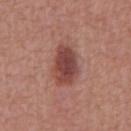Clinical impression:
Captured during whole-body skin photography for melanoma surveillance; the lesion was not biopsied.
Image and clinical context:
A 15 mm close-up tile from a total-body photography series done for melanoma screening. The recorded lesion diameter is about 5 mm. On the abdomen. Imaged with white-light lighting. A male patient, about 65 years old.